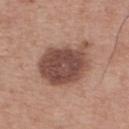Case summary:
- patient — male, aged approximately 65
- site — the upper back
- tile lighting — white-light illumination
- acquisition — 15 mm crop, total-body photography
- image-analysis metrics — an average lesion color of about L≈47 a*≈20 b*≈24 (CIELAB), about 15 CIELAB-L* units darker than the surrounding skin, and a normalized border contrast of about 10.5; a border-irregularity rating of about 2.5/10, internal color variation of about 3.5 on a 0–10 scale, and radial color variation of about 1; an automated nevus-likeness rating near 20 out of 100
- lesion diameter — ~6.5 mm (longest diameter)